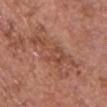Captured during whole-body skin photography for melanoma surveillance; the lesion was not biopsied.
A female patient, in their mid-60s.
Longest diameter approximately 8 mm.
On the chest.
A 15 mm close-up tile from a total-body photography series done for melanoma screening.
Captured under white-light illumination.